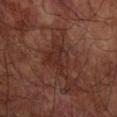workup: no biopsy performed (imaged during a skin exam); image: ~15 mm crop, total-body skin-cancer survey; lesion size: ≈4.5 mm; body site: the right forearm; patient: male, aged approximately 65; lighting: cross-polarized; image-analysis metrics: a detector confidence of about 90 out of 100 that the crop contains a lesion.